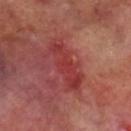{"image": {"source": "total-body photography crop", "field_of_view_mm": 15}, "site": "leg", "patient": {"sex": "male", "age_approx": 70}}